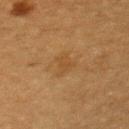notes=no biopsy performed (imaged during a skin exam); body site=the chest; automated lesion analysis=a lesion area of about 4.5 mm², a shape eccentricity near 0.5, and two-axis asymmetry of about 0.3; lighting=cross-polarized illumination; image=total-body-photography crop, ~15 mm field of view; subject=female, about 55 years old; lesion size=≈2.5 mm.